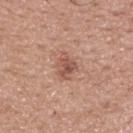| field | value |
|---|---|
| workup | catalogued during a skin exam; not biopsied |
| patient | male, approximately 40 years of age |
| diameter | ≈3.5 mm |
| tile lighting | white-light |
| acquisition | ~15 mm tile from a whole-body skin photo |
| site | the back |